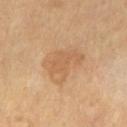Imaged during a routine full-body skin examination; the lesion was not biopsied and no histopathology is available.
A lesion tile, about 15 mm wide, cut from a 3D total-body photograph.
Located on the leg.
Captured under cross-polarized illumination.
A male patient, about 65 years old.